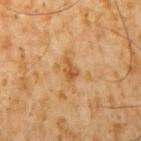A male patient approximately 60 years of age.
An algorithmic analysis of the crop reported a shape eccentricity near 0.8. It also reported a mean CIELAB color near L≈45 a*≈19 b*≈36 and a lesion–skin lightness drop of about 8. And it measured border irregularity of about 4 on a 0–10 scale. The software also gave a classifier nevus-likeness of about 0/100 and lesion-presence confidence of about 100/100.
Longest diameter approximately 2.5 mm.
A close-up tile cropped from a whole-body skin photograph, about 15 mm across.
Captured under cross-polarized illumination.
From the arm.